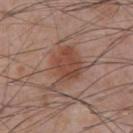Recorded during total-body skin imaging; not selected for excision or biopsy. Captured under white-light illumination. The recorded lesion diameter is about 5.5 mm. Located on the chest. The lesion-visualizer software estimated an eccentricity of roughly 0.65 and a symmetry-axis asymmetry near 0.35. The analysis additionally found a lesion–skin lightness drop of about 8 and a lesion-to-skin contrast of about 7 (normalized; higher = more distinct). The analysis additionally found a border-irregularity index near 4.5/10 and internal color variation of about 3.5 on a 0–10 scale. And it measured an automated nevus-likeness rating near 95 out of 100 and a detector confidence of about 100 out of 100 that the crop contains a lesion. A male patient roughly 60 years of age. A close-up tile cropped from a whole-body skin photograph, about 15 mm across.